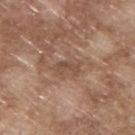Notes:
– biopsy status · imaged on a skin check; not biopsied
– patient · male, aged 63–67
– body site · the upper back
– imaging modality · 15 mm crop, total-body photography